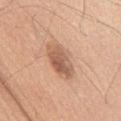Q: Was this lesion biopsied?
A: catalogued during a skin exam; not biopsied